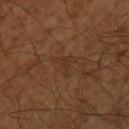Recorded during total-body skin imaging; not selected for excision or biopsy. A 15 mm close-up tile from a total-body photography series done for melanoma screening. On the upper back. A male patient aged 28 to 32.